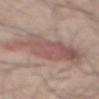workup: catalogued during a skin exam; not biopsied
subject: male, in their 50s
lighting: white-light
automated lesion analysis: a mean CIELAB color near L≈54 a*≈18 b*≈22 and a normalized lesion–skin contrast near 6.5
imaging modality: 15 mm crop, total-body photography
site: the back